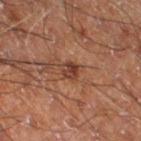This lesion was catalogued during total-body skin photography and was not selected for biopsy. On the right thigh. A male subject aged 58 to 62. A 15 mm close-up extracted from a 3D total-body photography capture.